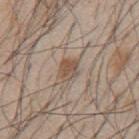{
  "biopsy_status": "not biopsied; imaged during a skin examination",
  "patient": {
    "sex": "male",
    "age_approx": 45
  },
  "automated_metrics": {
    "eccentricity": 0.6,
    "shape_asymmetry": 0.2,
    "lesion_detection_confidence_0_100": 100
  },
  "site": "back",
  "image": {
    "source": "total-body photography crop",
    "field_of_view_mm": 15
  },
  "lesion_size": {
    "long_diameter_mm_approx": 3.0
  }
}